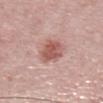The lesion was photographed on a routine skin check and not biopsied; there is no pathology result.
Captured under white-light illumination.
The patient is a male aged around 65.
Longest diameter approximately 4 mm.
Located on the mid back.
A roughly 15 mm field-of-view crop from a total-body skin photograph.